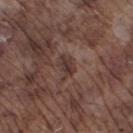Longest diameter approximately 3 mm.
Automated tile analysis of the lesion measured an average lesion color of about L≈35 a*≈17 b*≈20 (CIELAB). And it measured a border-irregularity index near 4/10, a color-variation rating of about 4/10, and peripheral color asymmetry of about 1.5.
A 15 mm crop from a total-body photograph taken for skin-cancer surveillance.
From the leg.
This is a white-light tile.
The subject is a male roughly 75 years of age.